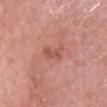Q: Was this lesion biopsied?
A: total-body-photography surveillance lesion; no biopsy
Q: How was this image acquired?
A: total-body-photography crop, ~15 mm field of view
Q: Lesion location?
A: the head or neck
Q: What lighting was used for the tile?
A: white-light
Q: What is the lesion's diameter?
A: ~3.5 mm (longest diameter)
Q: Automated lesion metrics?
A: a footprint of about 4 mm², a shape eccentricity near 0.9, and a shape-asymmetry score of about 0.4 (0 = symmetric)
Q: What are the patient's age and sex?
A: male, about 85 years old or older